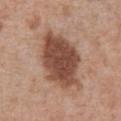This lesion was catalogued during total-body skin photography and was not selected for biopsy. From the abdomen. A male subject aged 68–72. This image is a 15 mm lesion crop taken from a total-body photograph. The total-body-photography lesion software estimated a lesion area of about 27 mm², an outline eccentricity of about 0.8 (0 = round, 1 = elongated), and two-axis asymmetry of about 0.2. The software also gave a nevus-likeness score of about 70/100 and lesion-presence confidence of about 100/100. Measured at roughly 8 mm in maximum diameter.